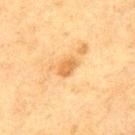Clinical impression:
No biopsy was performed on this lesion — it was imaged during a full skin examination and was not determined to be concerning.
Background:
A roughly 15 mm field-of-view crop from a total-body skin photograph. From the mid back. A male subject, aged 73–77.